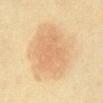notes — total-body-photography surveillance lesion; no biopsy
size — ≈7 mm
location — the abdomen
patient — female, about 60 years old
tile lighting — cross-polarized
automated lesion analysis — a lesion color around L≈64 a*≈17 b*≈36 in CIELAB and about 7 CIELAB-L* units darker than the surrounding skin
imaging modality — ~15 mm crop, total-body skin-cancer survey